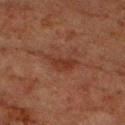{
  "biopsy_status": "not biopsied; imaged during a skin examination",
  "patient": {
    "sex": "male",
    "age_approx": 80
  },
  "image": {
    "source": "total-body photography crop",
    "field_of_view_mm": 15
  },
  "lesion_size": {
    "long_diameter_mm_approx": 4.0
  },
  "lighting": "cross-polarized",
  "site": "right upper arm"
}